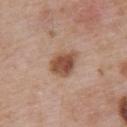| key | value |
|---|---|
| follow-up | catalogued during a skin exam; not biopsied |
| image | total-body-photography crop, ~15 mm field of view |
| lighting | white-light |
| size | about 4 mm |
| location | the upper back |
| patient | male, aged 53–57 |